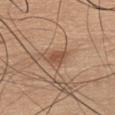The lesion was tiled from a total-body skin photograph and was not biopsied.
The subject is a male aged approximately 45.
Measured at roughly 2.5 mm in maximum diameter.
The lesion is on the chest.
Imaged with white-light lighting.
A 15 mm close-up extracted from a 3D total-body photography capture.